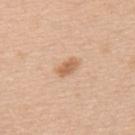No biopsy was performed on this lesion — it was imaged during a full skin examination and was not determined to be concerning. A male patient in their 50s. Automated image analysis of the tile measured a footprint of about 4 mm² and two-axis asymmetry of about 0.15. The software also gave an average lesion color of about L≈63 a*≈20 b*≈35 (CIELAB), about 11 CIELAB-L* units darker than the surrounding skin, and a normalized lesion–skin contrast near 7. The software also gave border irregularity of about 1.5 on a 0–10 scale, internal color variation of about 2 on a 0–10 scale, and a peripheral color-asymmetry measure near 0.5. The analysis additionally found a nevus-likeness score of about 80/100 and lesion-presence confidence of about 100/100. Imaged with white-light lighting. About 2.5 mm across. Located on the upper back. A 15 mm close-up tile from a total-body photography series done for melanoma screening.